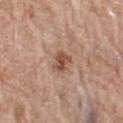<tbp_lesion>
  <biopsy_status>not biopsied; imaged during a skin examination</biopsy_status>
  <image>
    <source>total-body photography crop</source>
    <field_of_view_mm>15</field_of_view_mm>
  </image>
  <automated_metrics>
    <cielab_L>51</cielab_L>
    <cielab_a>20</cielab_a>
    <cielab_b>29</cielab_b>
    <vs_skin_darker_L>12.0</vs_skin_darker_L>
    <vs_skin_contrast_norm>8.0</vs_skin_contrast_norm>
    <color_variation_0_10>3.0</color_variation_0_10>
  </automated_metrics>
  <patient>
    <sex>female</sex>
    <age_approx>75</age_approx>
  </patient>
  <site>right thigh</site>
  <lighting>white-light</lighting>
</tbp_lesion>